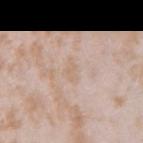The lesion was photographed on a routine skin check and not biopsied; there is no pathology result.
This is a white-light tile.
From the right upper arm.
Measured at roughly 2.5 mm in maximum diameter.
The lesion-visualizer software estimated a lesion color around L≈66 a*≈14 b*≈28 in CIELAB, a lesion–skin lightness drop of about 5, and a normalized border contrast of about 4.5. The analysis additionally found a border-irregularity index near 3.5/10, internal color variation of about 1 on a 0–10 scale, and peripheral color asymmetry of about 0.5.
A female subject approximately 25 years of age.
Cropped from a total-body skin-imaging series; the visible field is about 15 mm.